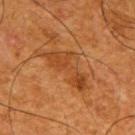Impression: The lesion was tiled from a total-body skin photograph and was not biopsied. Acquisition and patient details: The lesion's longest dimension is about 6.5 mm. From the upper back. This is a cross-polarized tile. The lesion-visualizer software estimated a lesion area of about 11 mm², an eccentricity of roughly 0.9, and two-axis asymmetry of about 0.6. A male patient, in their mid- to late 60s. Cropped from a whole-body photographic skin survey; the tile spans about 15 mm.